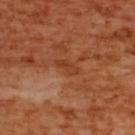* follow-up · catalogued during a skin exam; not biopsied
* anatomic site · the upper back
* image source · 15 mm crop, total-body photography
* patient · female, about 55 years old
* lesion size · ~3 mm (longest diameter)